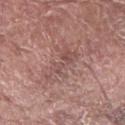Assessment:
This lesion was catalogued during total-body skin photography and was not selected for biopsy.
Image and clinical context:
Imaged with white-light lighting. Cropped from a whole-body photographic skin survey; the tile spans about 15 mm. Longest diameter approximately 5.5 mm. From the right forearm. A male patient, aged 73 to 77.